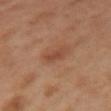- notes — total-body-photography surveillance lesion; no biopsy
- lighting — cross-polarized
- size — about 3 mm
- subject — female, aged around 40
- imaging modality — total-body-photography crop, ~15 mm field of view
- location — the left arm